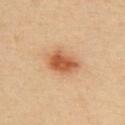Q: Is there a histopathology result?
A: total-body-photography surveillance lesion; no biopsy
Q: What is the imaging modality?
A: 15 mm crop, total-body photography
Q: Where on the body is the lesion?
A: the upper back
Q: What are the patient's age and sex?
A: male, about 40 years old